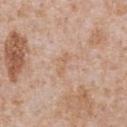| key | value |
|---|---|
| follow-up | no biopsy performed (imaged during a skin exam) |
| illumination | white-light illumination |
| imaging modality | total-body-photography crop, ~15 mm field of view |
| subject | male, approximately 65 years of age |
| lesion diameter | about 3 mm |
| anatomic site | the chest |